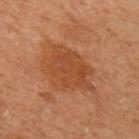Case summary:
• biopsy status: imaged on a skin check; not biopsied
• image: total-body-photography crop, ~15 mm field of view
• automated lesion analysis: a shape eccentricity near 0.75 and a symmetry-axis asymmetry near 0.25; an average lesion color of about L≈40 a*≈23 b*≈33 (CIELAB), roughly 7 lightness units darker than nearby skin, and a normalized border contrast of about 6; a classifier nevus-likeness of about 5/100 and a detector confidence of about 100 out of 100 that the crop contains a lesion
• site: the right arm
• subject: female, about 60 years old